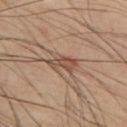  biopsy_status: not biopsied; imaged during a skin examination
  automated_metrics:
    border_irregularity_0_10: 4.0
    color_variation_0_10: 4.0
    peripheral_color_asymmetry: 1.5
    nevus_likeness_0_100: 0
    lesion_detection_confidence_0_100: 85
  patient:
    sex: male
    age_approx: 60
  lesion_size:
    long_diameter_mm_approx: 3.5
  lighting: cross-polarized
  image:
    source: total-body photography crop
    field_of_view_mm: 15
  site: back